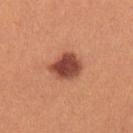Q: Was a biopsy performed?
A: no biopsy performed (imaged during a skin exam)
Q: Patient demographics?
A: female, aged 23 to 27
Q: What did automated image analysis measure?
A: border irregularity of about 2 on a 0–10 scale and internal color variation of about 3.5 on a 0–10 scale; a nevus-likeness score of about 90/100 and a detector confidence of about 100 out of 100 that the crop contains a lesion
Q: What lighting was used for the tile?
A: white-light illumination
Q: What is the lesion's diameter?
A: ~4 mm (longest diameter)
Q: Lesion location?
A: the left upper arm
Q: What is the imaging modality?
A: ~15 mm crop, total-body skin-cancer survey